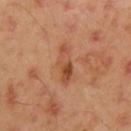follow-up=no biopsy performed (imaged during a skin exam); subject=male, approximately 45 years of age; anatomic site=the arm; imaging modality=15 mm crop, total-body photography.